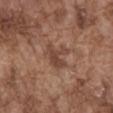Assessment: Recorded during total-body skin imaging; not selected for excision or biopsy. Background: Captured under white-light illumination. The lesion is on the abdomen. A region of skin cropped from a whole-body photographic capture, roughly 15 mm wide. A male subject, roughly 75 years of age. Approximately 3.5 mm at its widest.